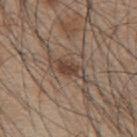- workup — no biopsy performed (imaged during a skin exam)
- patient — male, roughly 45 years of age
- automated metrics — a lesion–skin lightness drop of about 9 and a normalized lesion–skin contrast near 7.5
- location — the mid back
- imaging modality — ~15 mm tile from a whole-body skin photo
- lighting — white-light illumination